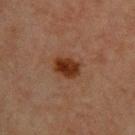Q: Was this lesion biopsied?
A: catalogued during a skin exam; not biopsied
Q: Where on the body is the lesion?
A: the back
Q: How was this image acquired?
A: 15 mm crop, total-body photography
Q: What lighting was used for the tile?
A: cross-polarized
Q: How large is the lesion?
A: about 3 mm
Q: What are the patient's age and sex?
A: male, aged around 60